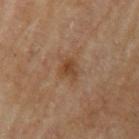Imaged during a routine full-body skin examination; the lesion was not biopsied and no histopathology is available.
A male patient, aged around 70.
Located on the left upper arm.
A 15 mm crop from a total-body photograph taken for skin-cancer surveillance.